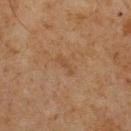Findings:
• biopsy status — total-body-photography surveillance lesion; no biopsy
• anatomic site — the chest
• TBP lesion metrics — an average lesion color of about L≈38 a*≈15 b*≈28 (CIELAB) and a normalized border contrast of about 4.5; a within-lesion color-variation index near 0/10 and radial color variation of about 0
• patient — male, in their mid-70s
• image source — ~15 mm crop, total-body skin-cancer survey
• lesion size — ≈2.5 mm